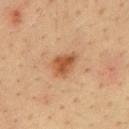follow-up = total-body-photography surveillance lesion; no biopsy | illumination = cross-polarized illumination | location = the mid back | acquisition = ~15 mm tile from a whole-body skin photo | lesion size = about 3.5 mm | patient = male, aged around 35.